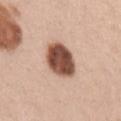biopsy status: total-body-photography surveillance lesion; no biopsy
TBP lesion metrics: a lesion color around L≈50 a*≈21 b*≈28 in CIELAB and a normalized border contrast of about 14; a border-irregularity rating of about 1.5/10 and radial color variation of about 1.5; a lesion-detection confidence of about 100/100
diameter: ≈4.5 mm
patient: female, aged approximately 40
image source: ~15 mm tile from a whole-body skin photo
lighting: white-light illumination
site: the left upper arm